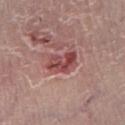Cropped from a whole-body photographic skin survey; the tile spans about 15 mm. Located on the right lower leg. A male patient, in their mid-50s.